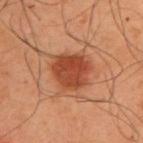No biopsy was performed on this lesion — it was imaged during a full skin examination and was not determined to be concerning.
The tile uses cross-polarized illumination.
A 15 mm crop from a total-body photograph taken for skin-cancer surveillance.
From the upper back.
The subject is a male roughly 55 years of age.